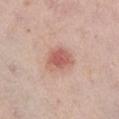{
  "biopsy_status": "not biopsied; imaged during a skin examination",
  "patient": {
    "sex": "female",
    "age_approx": 65
  },
  "automated_metrics": {
    "cielab_L": 59,
    "cielab_a": 25,
    "cielab_b": 27,
    "vs_skin_darker_L": 11.0,
    "vs_skin_contrast_norm": 7.5,
    "border_irregularity_0_10": 2.5,
    "color_variation_0_10": 3.5,
    "peripheral_color_asymmetry": 1.0
  },
  "lesion_size": {
    "long_diameter_mm_approx": 3.5
  },
  "lighting": "white-light",
  "site": "right lower leg",
  "image": {
    "source": "total-body photography crop",
    "field_of_view_mm": 15
  }
}